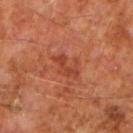The lesion was tiled from a total-body skin photograph and was not biopsied. On the right forearm. The lesion-visualizer software estimated an area of roughly 5.5 mm², a shape eccentricity near 0.75, and a symmetry-axis asymmetry near 0.35. And it measured a classifier nevus-likeness of about 0/100 and a lesion-detection confidence of about 100/100. The tile uses cross-polarized illumination. The patient is a male approximately 60 years of age. Cropped from a whole-body photographic skin survey; the tile spans about 15 mm.